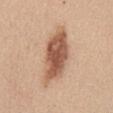Assessment: The lesion was tiled from a total-body skin photograph and was not biopsied. Context: From the abdomen. The tile uses white-light illumination. Longest diameter approximately 7 mm. The patient is a female aged around 35. Automated image analysis of the tile measured an eccentricity of roughly 0.85 and a shape-asymmetry score of about 0.2 (0 = symmetric). The software also gave peripheral color asymmetry of about 1.5. It also reported a classifier nevus-likeness of about 95/100 and a detector confidence of about 100 out of 100 that the crop contains a lesion. Cropped from a total-body skin-imaging series; the visible field is about 15 mm.